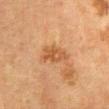{"biopsy_status": "not biopsied; imaged during a skin examination"}